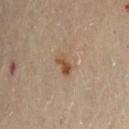A 15 mm crop from a total-body photograph taken for skin-cancer surveillance.
The lesion is located on the lower back.
The total-body-photography lesion software estimated an area of roughly 4.5 mm², an outline eccentricity of about 0.85 (0 = round, 1 = elongated), and a shape-asymmetry score of about 0.25 (0 = symmetric). The analysis additionally found an average lesion color of about L≈51 a*≈17 b*≈32 (CIELAB), about 9 CIELAB-L* units darker than the surrounding skin, and a lesion-to-skin contrast of about 8 (normalized; higher = more distinct). The software also gave border irregularity of about 3 on a 0–10 scale and a peripheral color-asymmetry measure near 2. The software also gave an automated nevus-likeness rating near 80 out of 100 and a detector confidence of about 100 out of 100 that the crop contains a lesion.
Measured at roughly 3 mm in maximum diameter.
A female subject approximately 60 years of age.